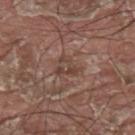Findings:
- notes · no biopsy performed (imaged during a skin exam)
- patient · male, aged 38–42
- image · ~15 mm crop, total-body skin-cancer survey
- illumination · white-light illumination
- site · the upper back
- diameter · about 3 mm